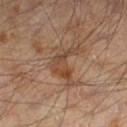notes = catalogued during a skin exam; not biopsied
imaging modality = 15 mm crop, total-body photography
TBP lesion metrics = a border-irregularity index near 7/10, internal color variation of about 5 on a 0–10 scale, and radial color variation of about 1
lesion diameter = ~4 mm (longest diameter)
patient = male, aged around 65
location = the left lower leg
illumination = cross-polarized illumination